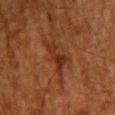{
  "biopsy_status": "not biopsied; imaged during a skin examination",
  "patient": {
    "sex": "male",
    "age_approx": 60
  },
  "automated_metrics": {
    "area_mm2_approx": 5.0,
    "eccentricity": 0.95,
    "cielab_L": 23,
    "cielab_a": 21,
    "cielab_b": 26,
    "vs_skin_darker_L": 6.0,
    "vs_skin_contrast_norm": 7.5,
    "border_irregularity_0_10": 7.0,
    "color_variation_0_10": 1.5,
    "peripheral_color_asymmetry": 0.5
  },
  "lighting": "cross-polarized",
  "image": {
    "source": "total-body photography crop",
    "field_of_view_mm": 15
  },
  "site": "chest"
}